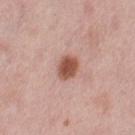Imaged during a routine full-body skin examination; the lesion was not biopsied and no histopathology is available. An algorithmic analysis of the crop reported internal color variation of about 2.5 on a 0–10 scale and radial color variation of about 0.5. A lesion tile, about 15 mm wide, cut from a 3D total-body photograph. The lesion is located on the leg. Measured at roughly 2.5 mm in maximum diameter. A female patient, aged around 50. This is a white-light tile.